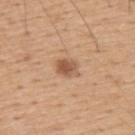{"biopsy_status": "not biopsied; imaged during a skin examination", "automated_metrics": {"shape_asymmetry": 0.25, "color_variation_0_10": 3.0, "peripheral_color_asymmetry": 1.0, "nevus_likeness_0_100": 90, "lesion_detection_confidence_0_100": 100}, "image": {"source": "total-body photography crop", "field_of_view_mm": 15}, "lighting": "white-light", "site": "upper back", "patient": {"sex": "male", "age_approx": 55}}